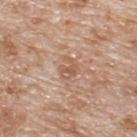Recorded during total-body skin imaging; not selected for excision or biopsy. A 15 mm close-up extracted from a 3D total-body photography capture. A male subject, roughly 80 years of age. On the back.